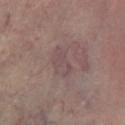Q: What is the imaging modality?
A: 15 mm crop, total-body photography
Q: What lighting was used for the tile?
A: cross-polarized illumination
Q: What is the lesion's diameter?
A: ≈4 mm
Q: Lesion location?
A: the left lower leg